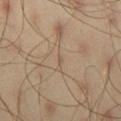  biopsy_status: not biopsied; imaged during a skin examination
  image:
    source: total-body photography crop
    field_of_view_mm: 15
  site: right thigh
  patient:
    sex: male
    age_approx: 45
  lighting: cross-polarized
  automated_metrics:
    color_variation_0_10: 0.0
    peripheral_color_asymmetry: 0.0
    nevus_likeness_0_100: 0